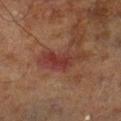follow-up: total-body-photography surveillance lesion; no biopsy
TBP lesion metrics: a nevus-likeness score of about 0/100 and a detector confidence of about 100 out of 100 that the crop contains a lesion
subject: male, in their 70s
location: the right lower leg
acquisition: 15 mm crop, total-body photography
diameter: ~5.5 mm (longest diameter)